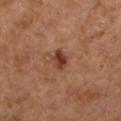The lesion was photographed on a routine skin check and not biopsied; there is no pathology result.
The lesion is located on the left lower leg.
Automated tile analysis of the lesion measured a peripheral color-asymmetry measure near 1.
Approximately 3 mm at its widest.
A lesion tile, about 15 mm wide, cut from a 3D total-body photograph.
Imaged with cross-polarized lighting.
A female subject, about 65 years old.